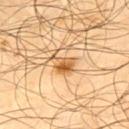A 15 mm close-up tile from a total-body photography series done for melanoma screening. From the upper back. A male patient, roughly 65 years of age. About 3 mm across. The lesion-visualizer software estimated an automated nevus-likeness rating near 95 out of 100.